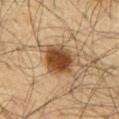| field | value |
|---|---|
| workup | total-body-photography surveillance lesion; no biopsy |
| location | the abdomen |
| subject | male, approximately 65 years of age |
| image | ~15 mm tile from a whole-body skin photo |
| illumination | cross-polarized |
| automated metrics | a normalized border contrast of about 12; a border-irregularity index near 2/10, a color-variation rating of about 4.5/10, and radial color variation of about 1.5; a classifier nevus-likeness of about 100/100 |